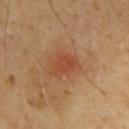Impression: Imaged during a routine full-body skin examination; the lesion was not biopsied and no histopathology is available. Image and clinical context: The subject is a male aged around 65. A lesion tile, about 15 mm wide, cut from a 3D total-body photograph. The lesion is located on the chest. Automated tile analysis of the lesion measured border irregularity of about 3.5 on a 0–10 scale and a peripheral color-asymmetry measure near 1. The analysis additionally found an automated nevus-likeness rating near 0 out of 100 and lesion-presence confidence of about 100/100. Captured under cross-polarized illumination.